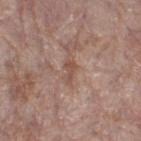workup — no biopsy performed (imaged during a skin exam)
lighting — white-light illumination
automated metrics — a footprint of about 2.5 mm² and a symmetry-axis asymmetry near 0.6; internal color variation of about 0.5 on a 0–10 scale and a peripheral color-asymmetry measure near 0; a classifier nevus-likeness of about 0/100 and a lesion-detection confidence of about 95/100
site — the right thigh
patient — female, roughly 85 years of age or older
image — 15 mm crop, total-body photography